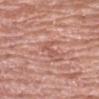Assessment:
The lesion was photographed on a routine skin check and not biopsied; there is no pathology result.
Background:
From the right upper arm. A female patient, in their 60s. A lesion tile, about 15 mm wide, cut from a 3D total-body photograph.